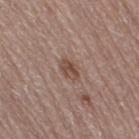workup=catalogued during a skin exam; not biopsied
imaging modality=total-body-photography crop, ~15 mm field of view
subject=female, roughly 65 years of age
body site=the right thigh